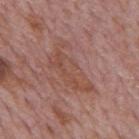follow-up = total-body-photography surveillance lesion; no biopsy
illumination = white-light
patient = male, aged 73 to 77
image source = 15 mm crop, total-body photography
location = the mid back